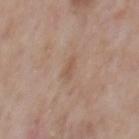The lesion was tiled from a total-body skin photograph and was not biopsied. The lesion's longest dimension is about 2.5 mm. The tile uses white-light illumination. An algorithmic analysis of the crop reported internal color variation of about 1 on a 0–10 scale and a peripheral color-asymmetry measure near 0. And it measured a detector confidence of about 100 out of 100 that the crop contains a lesion. A male patient about 80 years old. A 15 mm close-up tile from a total-body photography series done for melanoma screening. The lesion is on the chest.